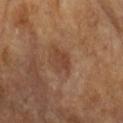Clinical impression: This lesion was catalogued during total-body skin photography and was not selected for biopsy. Image and clinical context: A 15 mm crop from a total-body photograph taken for skin-cancer surveillance. Automated image analysis of the tile measured a lesion area of about 4.5 mm², a shape eccentricity near 0.7, and a symmetry-axis asymmetry near 0.3. The analysis additionally found a border-irregularity index near 3/10, a color-variation rating of about 2.5/10, and radial color variation of about 1. It also reported an automated nevus-likeness rating near 5 out of 100 and a detector confidence of about 100 out of 100 that the crop contains a lesion. About 3 mm across. From the left forearm. The subject is a female roughly 75 years of age. Captured under cross-polarized illumination.